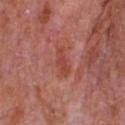biopsy status=catalogued during a skin exam; not biopsied | body site=the chest | subject=male, roughly 65 years of age | image source=~15 mm crop, total-body skin-cancer survey.